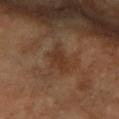automated metrics: a classifier nevus-likeness of about 0/100; diameter: about 4 mm; subject: female, aged 68–72; illumination: cross-polarized illumination; imaging modality: total-body-photography crop, ~15 mm field of view; body site: the left forearm.